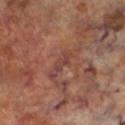{
  "biopsy_status": "not biopsied; imaged during a skin examination",
  "patient": {
    "sex": "male",
    "age_approx": 70
  },
  "site": "left lower leg",
  "automated_metrics": {
    "area_mm2_approx": 2.5,
    "eccentricity": 0.9,
    "shape_asymmetry": 0.35,
    "cielab_L": 40,
    "cielab_a": 22,
    "cielab_b": 24,
    "vs_skin_darker_L": 6.0,
    "vs_skin_contrast_norm": 6.0
  },
  "lesion_size": {
    "long_diameter_mm_approx": 3.0
  },
  "lighting": "cross-polarized",
  "image": {
    "source": "total-body photography crop",
    "field_of_view_mm": 15
  }
}